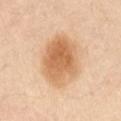  biopsy_status: not biopsied; imaged during a skin examination
  automated_metrics:
    cielab_L: 66
    cielab_a: 21
    cielab_b: 39
    border_irregularity_0_10: 2.0
    color_variation_0_10: 5.0
    nevus_likeness_0_100: 95
    lesion_detection_confidence_0_100: 100
  image:
    source: total-body photography crop
    field_of_view_mm: 15
  lighting: cross-polarized
  site: abdomen
  lesion_size:
    long_diameter_mm_approx: 6.5